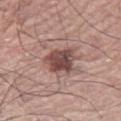Clinical impression: No biopsy was performed on this lesion — it was imaged during a full skin examination and was not determined to be concerning. Clinical summary: From the left leg. A male subject, in their 70s. This image is a 15 mm lesion crop taken from a total-body photograph.